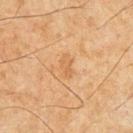follow-up=total-body-photography surveillance lesion; no biopsy
subject=male, about 65 years old
imaging modality=total-body-photography crop, ~15 mm field of view
TBP lesion metrics=a classifier nevus-likeness of about 0/100 and a detector confidence of about 100 out of 100 that the crop contains a lesion
body site=the chest